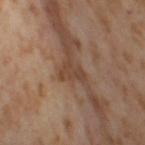{
  "lighting": "cross-polarized",
  "lesion_size": {
    "long_diameter_mm_approx": 3.0
  },
  "site": "right thigh",
  "patient": {
    "sex": "female",
    "age_approx": 55
  },
  "image": {
    "source": "total-body photography crop",
    "field_of_view_mm": 15
  }
}